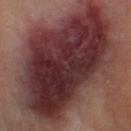{
  "biopsy_status": "not biopsied; imaged during a skin examination",
  "image": {
    "source": "total-body photography crop",
    "field_of_view_mm": 15
  },
  "automated_metrics": {
    "cielab_L": 33,
    "cielab_a": 22,
    "cielab_b": 18,
    "vs_skin_darker_L": 15.0,
    "vs_skin_contrast_norm": 13.5,
    "border_irregularity_0_10": 5.5,
    "color_variation_0_10": 9.5,
    "peripheral_color_asymmetry": 3.0,
    "nevus_likeness_0_100": 0
  },
  "site": "right thigh",
  "lighting": "cross-polarized",
  "patient": {
    "sex": "male",
    "age_approx": 65
  },
  "lesion_size": {
    "long_diameter_mm_approx": 18.5
  }
}